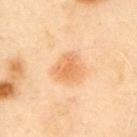* follow-up — total-body-photography surveillance lesion; no biopsy
* lighting — cross-polarized illumination
* automated metrics — an average lesion color of about L≈56 a*≈21 b*≈36 (CIELAB), about 8 CIELAB-L* units darker than the surrounding skin, and a normalized lesion–skin contrast near 6.5; an automated nevus-likeness rating near 95 out of 100
* acquisition — ~15 mm crop, total-body skin-cancer survey
* subject — male, aged 53–57
* size — ≈3 mm
* body site — the left upper arm